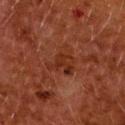Q: What is the imaging modality?
A: total-body-photography crop, ~15 mm field of view
Q: Where on the body is the lesion?
A: the back
Q: Patient demographics?
A: female, about 50 years old
Q: Lesion size?
A: ≈4 mm
Q: How was the tile lit?
A: cross-polarized illumination